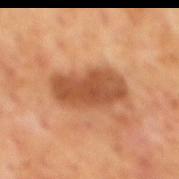No biopsy was performed on this lesion — it was imaged during a full skin examination and was not determined to be concerning. Located on the mid back. A 15 mm crop from a total-body photograph taken for skin-cancer surveillance. A male subject aged around 70.